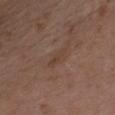{
  "biopsy_status": "not biopsied; imaged during a skin examination",
  "site": "chest",
  "patient": {
    "sex": "female",
    "age_approx": 35
  },
  "image": {
    "source": "total-body photography crop",
    "field_of_view_mm": 15
  },
  "lesion_size": {
    "long_diameter_mm_approx": 3.0
  },
  "lighting": "white-light"
}